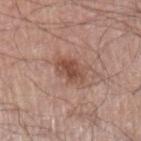Part of a total-body skin-imaging series; this lesion was reviewed on a skin check and was not flagged for biopsy.
A 15 mm crop from a total-body photograph taken for skin-cancer surveillance.
The lesion is on the left upper arm.
A male patient, aged 68 to 72.